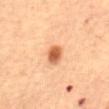{"biopsy_status": "not biopsied; imaged during a skin examination", "lighting": "cross-polarized", "image": {"source": "total-body photography crop", "field_of_view_mm": 15}, "site": "abdomen", "automated_metrics": {"eccentricity": 0.65, "shape_asymmetry": 0.15, "cielab_L": 54, "cielab_a": 25, "cielab_b": 37, "vs_skin_contrast_norm": 10.0, "color_variation_0_10": 4.5}, "patient": {"sex": "female", "age_approx": 60}}